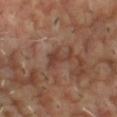Part of a total-body skin-imaging series; this lesion was reviewed on a skin check and was not flagged for biopsy.
Automated image analysis of the tile measured an outline eccentricity of about 0.9 (0 = round, 1 = elongated) and a symmetry-axis asymmetry near 0.65. The analysis additionally found about 7 CIELAB-L* units darker than the surrounding skin and a normalized lesion–skin contrast near 6.5. The analysis additionally found a border-irregularity index near 7.5/10, a within-lesion color-variation index near 1.5/10, and peripheral color asymmetry of about 0.5.
The subject is a male in their mid-50s.
About 4 mm across.
Captured under cross-polarized illumination.
A close-up tile cropped from a whole-body skin photograph, about 15 mm across.
Located on the chest.